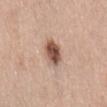{"biopsy_status": "not biopsied; imaged during a skin examination", "patient": {"sex": "female", "age_approx": 65}, "site": "lower back", "image": {"source": "total-body photography crop", "field_of_view_mm": 15}}